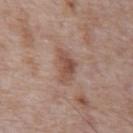Assessment: Captured during whole-body skin photography for melanoma surveillance; the lesion was not biopsied. Context: Imaged with white-light lighting. A male patient aged 68–72. The recorded lesion diameter is about 4 mm. A close-up tile cropped from a whole-body skin photograph, about 15 mm across. The lesion is on the abdomen.